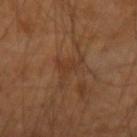Captured during whole-body skin photography for melanoma surveillance; the lesion was not biopsied. Located on the left arm. A roughly 15 mm field-of-view crop from a total-body skin photograph. Automated tile analysis of the lesion measured border irregularity of about 8.5 on a 0–10 scale and a color-variation rating of about 2.5/10. A male subject roughly 50 years of age. This is a cross-polarized tile.